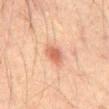This is a cross-polarized tile.
A 15 mm close-up tile from a total-body photography series done for melanoma screening.
The lesion's longest dimension is about 2.5 mm.
A male patient aged 43–47.
Located on the abdomen.